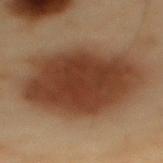Findings:
– notes: catalogued during a skin exam; not biopsied
– patient: male, about 55 years old
– body site: the mid back
– imaging modality: ~15 mm tile from a whole-body skin photo